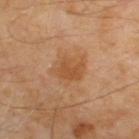{
  "biopsy_status": "not biopsied; imaged during a skin examination",
  "automated_metrics": {
    "eccentricity": 0.65,
    "shape_asymmetry": 0.25,
    "nevus_likeness_0_100": 15,
    "lesion_detection_confidence_0_100": 100
  },
  "image": {
    "source": "total-body photography crop",
    "field_of_view_mm": 15
  },
  "site": "back",
  "lesion_size": {
    "long_diameter_mm_approx": 4.0
  },
  "patient": {
    "sex": "male",
    "age_approx": 65
  },
  "lighting": "cross-polarized"
}